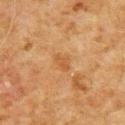Impression: Recorded during total-body skin imaging; not selected for excision or biopsy. Background: From the back. A male patient, about 80 years old. A 15 mm crop from a total-body photograph taken for skin-cancer surveillance.